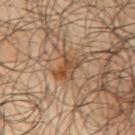No biopsy was performed on this lesion — it was imaged during a full skin examination and was not determined to be concerning.
The tile uses cross-polarized illumination.
The total-body-photography lesion software estimated a mean CIELAB color near L≈45 a*≈18 b*≈32 and about 9 CIELAB-L* units darker than the surrounding skin. And it measured a border-irregularity index near 4/10 and radial color variation of about 1.5. The software also gave a lesion-detection confidence of about 95/100.
The patient is a male in their mid-60s.
The lesion is located on the arm.
The lesion's longest dimension is about 3.5 mm.
A 15 mm close-up tile from a total-body photography series done for melanoma screening.